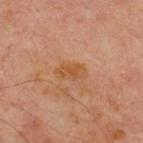Q: What is the imaging modality?
A: ~15 mm tile from a whole-body skin photo
Q: What are the patient's age and sex?
A: male, aged 68 to 72
Q: Lesion location?
A: the front of the torso